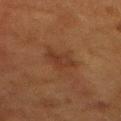Clinical impression: No biopsy was performed on this lesion — it was imaged during a full skin examination and was not determined to be concerning. Context: About 3.5 mm across. The lesion is on the mid back. Imaged with cross-polarized lighting. A female patient, aged 48 to 52. A lesion tile, about 15 mm wide, cut from a 3D total-body photograph.